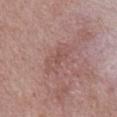Q: Was this lesion biopsied?
A: total-body-photography surveillance lesion; no biopsy
Q: Where on the body is the lesion?
A: the chest
Q: What is the imaging modality?
A: total-body-photography crop, ~15 mm field of view
Q: What did automated image analysis measure?
A: a footprint of about 1.5 mm², an eccentricity of roughly 0.8, and a symmetry-axis asymmetry near 0.3; border irregularity of about 2.5 on a 0–10 scale, a within-lesion color-variation index near 0/10, and radial color variation of about 0
Q: What lighting was used for the tile?
A: white-light illumination
Q: What are the patient's age and sex?
A: male, in their mid- to late 60s
Q: What is the lesion's diameter?
A: ≈1.5 mm